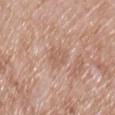workup — imaged on a skin check; not biopsied
body site — the chest
image — 15 mm crop, total-body photography
TBP lesion metrics — a border-irregularity rating of about 3/10 and a color-variation rating of about 2/10; a classifier nevus-likeness of about 0/100 and a lesion-detection confidence of about 100/100
patient — male, roughly 50 years of age